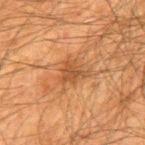The subject is a male approximately 60 years of age. Automated image analysis of the tile measured an area of roughly 5.5 mm², a shape eccentricity near 0.55, and two-axis asymmetry of about 0.45. The analysis additionally found a border-irregularity rating of about 4.5/10, a color-variation rating of about 2/10, and a peripheral color-asymmetry measure near 0.5. The analysis additionally found a nevus-likeness score of about 0/100. The recorded lesion diameter is about 3 mm. A 15 mm close-up extracted from a 3D total-body photography capture. Captured under cross-polarized illumination. From the upper back.